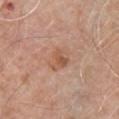<tbp_lesion>
  <biopsy_status>not biopsied; imaged during a skin examination</biopsy_status>
  <lighting>white-light</lighting>
  <lesion_size>
    <long_diameter_mm_approx>3.5</long_diameter_mm_approx>
  </lesion_size>
  <site>chest</site>
  <image>
    <source>total-body photography crop</source>
    <field_of_view_mm>15</field_of_view_mm>
  </image>
  <automated_metrics>
    <eccentricity>0.6</eccentricity>
    <shape_asymmetry>0.2</shape_asymmetry>
    <cielab_L>56</cielab_L>
    <cielab_a>21</cielab_a>
    <cielab_b>31</cielab_b>
    <vs_skin_darker_L>7.0</vs_skin_darker_L>
    <vs_skin_contrast_norm>6.0</vs_skin_contrast_norm>
    <nevus_likeness_0_100>0</nevus_likeness_0_100>
  </automated_metrics>
  <patient>
    <sex>male</sex>
    <age_approx>80</age_approx>
  </patient>
</tbp_lesion>